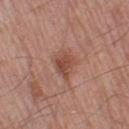Image and clinical context: On the left thigh. Imaged with white-light lighting. Measured at roughly 3 mm in maximum diameter. A close-up tile cropped from a whole-body skin photograph, about 15 mm across. The subject is a male aged around 55.